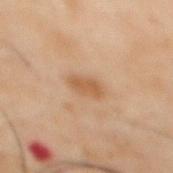The lesion was tiled from a total-body skin photograph and was not biopsied.